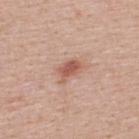Cropped from a total-body skin-imaging series; the visible field is about 15 mm.
The patient is a male roughly 40 years of age.
Longest diameter approximately 3 mm.
Automated image analysis of the tile measured an eccentricity of roughly 0.8 and a shape-asymmetry score of about 0.3 (0 = symmetric). The analysis additionally found a mean CIELAB color near L≈56 a*≈24 b*≈28. It also reported a border-irregularity rating of about 3.5/10, a color-variation rating of about 2/10, and radial color variation of about 0.5.
From the upper back.